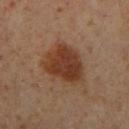Impression: The lesion was photographed on a routine skin check and not biopsied; there is no pathology result. Acquisition and patient details: The lesion is on the right lower leg. Captured under cross-polarized illumination. A lesion tile, about 15 mm wide, cut from a 3D total-body photograph. Longest diameter approximately 5.5 mm. A male subject, aged 58–62.